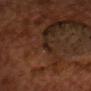Recorded during total-body skin imaging; not selected for excision or biopsy. Captured under cross-polarized illumination. An algorithmic analysis of the crop reported a footprint of about 1 mm², a shape eccentricity near 0.85, and a shape-asymmetry score of about 0.4 (0 = symmetric). The software also gave a border-irregularity index near 3.5/10. From the head or neck. Cropped from a total-body skin-imaging series; the visible field is about 15 mm. A male subject aged 53–57.